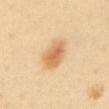{"biopsy_status": "not biopsied; imaged during a skin examination", "site": "abdomen", "lesion_size": {"long_diameter_mm_approx": 4.0}, "patient": {"sex": "female", "age_approx": 40}, "image": {"source": "total-body photography crop", "field_of_view_mm": 15}, "lighting": "cross-polarized"}